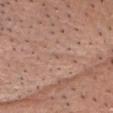Clinical impression:
Captured during whole-body skin photography for melanoma surveillance; the lesion was not biopsied.
Background:
The lesion-visualizer software estimated a footprint of about 1.5 mm², an eccentricity of roughly 0.9, and a symmetry-axis asymmetry near 0.35. And it measured an automated nevus-likeness rating near 0 out of 100 and a lesion-detection confidence of about 40/100. Located on the head or neck. A lesion tile, about 15 mm wide, cut from a 3D total-body photograph. A male subject about 60 years old. Captured under white-light illumination. Longest diameter approximately 2 mm.